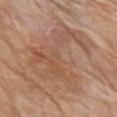Imaged during a routine full-body skin examination; the lesion was not biopsied and no histopathology is available.
Located on the left upper arm.
Cropped from a whole-body photographic skin survey; the tile spans about 15 mm.
The total-body-photography lesion software estimated an area of roughly 35 mm² and two-axis asymmetry of about 0.65. And it measured a mean CIELAB color near L≈54 a*≈20 b*≈30, a lesion–skin lightness drop of about 7, and a normalized lesion–skin contrast near 5.
Approximately 10 mm at its widest.
This is a white-light tile.
A male subject about 80 years old.